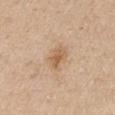biopsy status: imaged on a skin check; not biopsied | subject: male, aged 73–77 | TBP lesion metrics: a footprint of about 5 mm², an eccentricity of roughly 0.75, and a shape-asymmetry score of about 0.2 (0 = symmetric); a lesion color around L≈60 a*≈18 b*≈34 in CIELAB, a lesion–skin lightness drop of about 9, and a normalized lesion–skin contrast near 6.5; a border-irregularity rating of about 2.5/10, a color-variation rating of about 3/10, and a peripheral color-asymmetry measure near 1; an automated nevus-likeness rating near 30 out of 100 and a lesion-detection confidence of about 100/100 | image: ~15 mm tile from a whole-body skin photo | lesion diameter: ~3 mm (longest diameter) | location: the right upper arm.